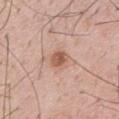Q: Was a biopsy performed?
A: imaged on a skin check; not biopsied
Q: What is the anatomic site?
A: the upper back
Q: Who is the patient?
A: male, aged around 55
Q: What is the imaging modality?
A: 15 mm crop, total-body photography
Q: What lighting was used for the tile?
A: white-light
Q: What did automated image analysis measure?
A: a border-irregularity rating of about 2/10, a within-lesion color-variation index near 4/10, and a peripheral color-asymmetry measure near 1.5; a detector confidence of about 100 out of 100 that the crop contains a lesion